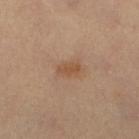Imaged during a routine full-body skin examination; the lesion was not biopsied and no histopathology is available. The subject is a female aged 43–47. The lesion is located on the right lower leg. A 15 mm close-up tile from a total-body photography series done for melanoma screening. This is a cross-polarized tile.